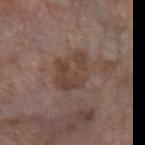| key | value |
|---|---|
| workup | imaged on a skin check; not biopsied |
| TBP lesion metrics | a mean CIELAB color near L≈42 a*≈16 b*≈23, a lesion–skin lightness drop of about 7, and a lesion-to-skin contrast of about 6.5 (normalized; higher = more distinct); border irregularity of about 2.5 on a 0–10 scale and a peripheral color-asymmetry measure near 1; a nevus-likeness score of about 0/100 and a lesion-detection confidence of about 100/100 |
| imaging modality | total-body-photography crop, ~15 mm field of view |
| tile lighting | white-light |
| subject | male, in their 60s |
| body site | the left forearm |